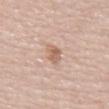No biopsy was performed on this lesion — it was imaged during a full skin examination and was not determined to be concerning. A close-up tile cropped from a whole-body skin photograph, about 15 mm across. A male patient, in their mid- to late 70s. This is a white-light tile. The lesion's longest dimension is about 2.5 mm. Located on the mid back. Automated tile analysis of the lesion measured a symmetry-axis asymmetry near 0.2. The software also gave a border-irregularity rating of about 2.5/10.